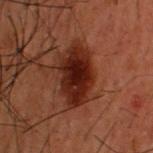Clinical impression:
Imaged during a routine full-body skin examination; the lesion was not biopsied and no histopathology is available.
Image and clinical context:
On the upper back. Imaged with cross-polarized lighting. A male patient, roughly 50 years of age. Longest diameter approximately 6 mm. A roughly 15 mm field-of-view crop from a total-body skin photograph.